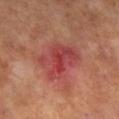notes = catalogued during a skin exam; not biopsied | lesion size = about 6 mm | patient = male, in their mid- to late 60s | imaging modality = total-body-photography crop, ~15 mm field of view | lighting = cross-polarized illumination.